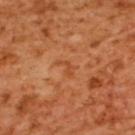Impression:
Recorded during total-body skin imaging; not selected for excision or biopsy.
Context:
A female patient, roughly 55 years of age. The lesion is on the upper back. Cropped from a total-body skin-imaging series; the visible field is about 15 mm. Approximately 2.5 mm at its widest. Imaged with cross-polarized lighting. Automated image analysis of the tile measured an area of roughly 2.5 mm², an outline eccentricity of about 0.85 (0 = round, 1 = elongated), and two-axis asymmetry of about 0.6.